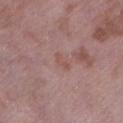This lesion was catalogued during total-body skin photography and was not selected for biopsy. A female patient about 70 years old. Cropped from a total-body skin-imaging series; the visible field is about 15 mm. About 2.5 mm across. This is a white-light tile. Automated tile analysis of the lesion measured an outline eccentricity of about 0.9 (0 = round, 1 = elongated) and two-axis asymmetry of about 0.3. The software also gave a lesion–skin lightness drop of about 6 and a normalized lesion–skin contrast near 5. It also reported a border-irregularity index near 3.5/10, internal color variation of about 0 on a 0–10 scale, and peripheral color asymmetry of about 0. The lesion is located on the left lower leg.